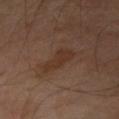Part of a total-body skin-imaging series; this lesion was reviewed on a skin check and was not flagged for biopsy.
A roughly 15 mm field-of-view crop from a total-body skin photograph.
The lesion is on the left forearm.
A male patient about 65 years old.